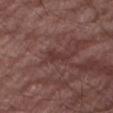Impression:
Part of a total-body skin-imaging series; this lesion was reviewed on a skin check and was not flagged for biopsy.
Clinical summary:
Located on the left leg. Cropped from a total-body skin-imaging series; the visible field is about 15 mm. A male subject, in their 80s.